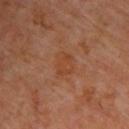Acquisition and patient details:
The lesion-visualizer software estimated a lesion color around L≈42 a*≈24 b*≈33 in CIELAB, roughly 5 lightness units darker than nearby skin, and a normalized lesion–skin contrast near 5. And it measured a border-irregularity index near 3/10, a within-lesion color-variation index near 2.5/10, and radial color variation of about 1. It also reported a nevus-likeness score of about 0/100 and a detector confidence of about 100 out of 100 that the crop contains a lesion. A male subject, roughly 60 years of age. Approximately 3 mm at its widest. The lesion is on the upper back. A 15 mm crop from a total-body photograph taken for skin-cancer surveillance. This is a cross-polarized tile.